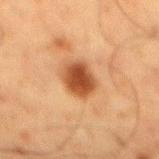  image:
    source: total-body photography crop
    field_of_view_mm: 15
  patient:
    sex: male
    age_approx: 60
  lesion_size:
    long_diameter_mm_approx: 4.0
  site: mid back